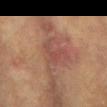workup: total-body-photography surveillance lesion; no biopsy | lesion size: about 3 mm | image source: ~15 mm tile from a whole-body skin photo | automated metrics: a footprint of about 4 mm², an eccentricity of roughly 0.75, and a symmetry-axis asymmetry near 0.65; border irregularity of about 7 on a 0–10 scale and radial color variation of about 0.5 | body site: the arm | patient: female, about 80 years old | lighting: cross-polarized illumination.